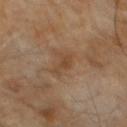Part of a total-body skin-imaging series; this lesion was reviewed on a skin check and was not flagged for biopsy. Captured under cross-polarized illumination. A male patient, roughly 60 years of age. The lesion is located on the abdomen. A close-up tile cropped from a whole-body skin photograph, about 15 mm across.